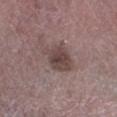Clinical impression: No biopsy was performed on this lesion — it was imaged during a full skin examination and was not determined to be concerning. Background: Cropped from a total-body skin-imaging series; the visible field is about 15 mm. The lesion is located on the left lower leg. A male patient roughly 75 years of age.